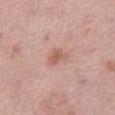Findings:
- notes: total-body-photography surveillance lesion; no biopsy
- patient: male, aged around 55
- image source: 15 mm crop, total-body photography
- TBP lesion metrics: a lesion area of about 4 mm², an outline eccentricity of about 0.75 (0 = round, 1 = elongated), and a symmetry-axis asymmetry near 0.4; a lesion color around L≈59 a*≈22 b*≈28 in CIELAB and a lesion–skin lightness drop of about 9
- tile lighting: white-light illumination
- size: ≈3 mm
- site: the right thigh